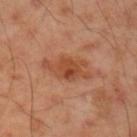<record>
  <biopsy_status>not biopsied; imaged during a skin examination</biopsy_status>
  <lesion_size>
    <long_diameter_mm_approx>5.5</long_diameter_mm_approx>
  </lesion_size>
  <site>left upper arm</site>
  <image>
    <source>total-body photography crop</source>
    <field_of_view_mm>15</field_of_view_mm>
  </image>
  <patient>
    <sex>male</sex>
    <age_approx>45</age_approx>
  </patient>
  <lighting>cross-polarized</lighting>
</record>